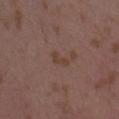Captured during whole-body skin photography for melanoma surveillance; the lesion was not biopsied. On the right forearm. Captured under white-light illumination. About 2.5 mm across. Cropped from a whole-body photographic skin survey; the tile spans about 15 mm. The patient is a female in their mid- to late 30s.